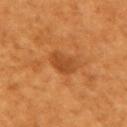Q: Is there a histopathology result?
A: imaged on a skin check; not biopsied
Q: Who is the patient?
A: female, aged 53–57
Q: How large is the lesion?
A: ≈3.5 mm
Q: What kind of image is this?
A: total-body-photography crop, ~15 mm field of view
Q: Lesion location?
A: the left upper arm